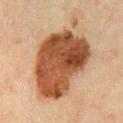Q: Was this lesion biopsied?
A: imaged on a skin check; not biopsied
Q: What did automated image analysis measure?
A: an outline eccentricity of about 0.8 (0 = round, 1 = elongated) and a symmetry-axis asymmetry near 0.25; a border-irregularity rating of about 3/10, internal color variation of about 8 on a 0–10 scale, and peripheral color asymmetry of about 3; a nevus-likeness score of about 90/100 and lesion-presence confidence of about 100/100
Q: Where on the body is the lesion?
A: the chest
Q: How was the tile lit?
A: cross-polarized
Q: How was this image acquired?
A: ~15 mm tile from a whole-body skin photo
Q: What is the lesion's diameter?
A: ~11.5 mm (longest diameter)
Q: Who is the patient?
A: male, about 70 years old